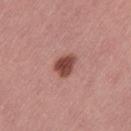Background: A close-up tile cropped from a whole-body skin photograph, about 15 mm across. The lesion is on the left thigh. Automated tile analysis of the lesion measured a lesion–skin lightness drop of about 16 and a normalized lesion–skin contrast near 11. The analysis additionally found border irregularity of about 2.5 on a 0–10 scale, a color-variation rating of about 2.5/10, and peripheral color asymmetry of about 0.5. This is a white-light tile. A female subject, aged 53–57.